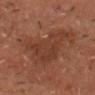Captured during whole-body skin photography for melanoma surveillance; the lesion was not biopsied.
The patient is a male in their mid- to late 60s.
Imaged with cross-polarized lighting.
The lesion's longest dimension is about 6.5 mm.
The lesion is on the head or neck.
A region of skin cropped from a whole-body photographic capture, roughly 15 mm wide.